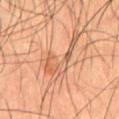Q: What are the patient's age and sex?
A: male, aged 53 to 57
Q: What is the imaging modality?
A: ~15 mm crop, total-body skin-cancer survey
Q: What is the lesion's diameter?
A: ≈7 mm
Q: Lesion location?
A: the abdomen
Q: Automated lesion metrics?
A: a footprint of about 12 mm², a shape eccentricity near 0.9, and two-axis asymmetry of about 0.75; a lesion color around L≈57 a*≈22 b*≈33 in CIELAB, a lesion–skin lightness drop of about 8, and a normalized border contrast of about 5.5; a border-irregularity rating of about 10/10, a within-lesion color-variation index near 4.5/10, and peripheral color asymmetry of about 1.5; lesion-presence confidence of about 85/100